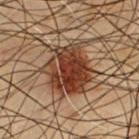Part of a total-body skin-imaging series; this lesion was reviewed on a skin check and was not flagged for biopsy. The lesion is on the front of the torso. Imaged with cross-polarized lighting. The lesion-visualizer software estimated a footprint of about 24 mm², a shape eccentricity near 0.55, and a shape-asymmetry score of about 0.25 (0 = symmetric). The recorded lesion diameter is about 6.5 mm. Cropped from a total-body skin-imaging series; the visible field is about 15 mm. A male patient, aged around 55.